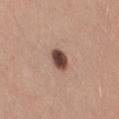Impression: The lesion was photographed on a routine skin check and not biopsied; there is no pathology result. Image and clinical context: Located on the right thigh. The lesion's longest dimension is about 3 mm. A lesion tile, about 15 mm wide, cut from a 3D total-body photograph. A female subject, about 45 years old.